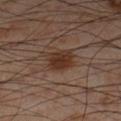{
  "biopsy_status": "not biopsied; imaged during a skin examination",
  "lesion_size": {
    "long_diameter_mm_approx": 3.5
  },
  "lighting": "cross-polarized",
  "image": {
    "source": "total-body photography crop",
    "field_of_view_mm": 15
  },
  "site": "leg",
  "patient": {
    "sex": "male",
    "age_approx": 55
  }
}